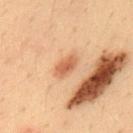Q: Is there a histopathology result?
A: imaged on a skin check; not biopsied
Q: What is the anatomic site?
A: the upper back
Q: What are the patient's age and sex?
A: male, about 35 years old
Q: Illumination type?
A: cross-polarized illumination
Q: How was this image acquired?
A: ~15 mm crop, total-body skin-cancer survey
Q: What is the lesion's diameter?
A: ≈3.5 mm